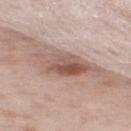Clinical impression: The lesion was photographed on a routine skin check and not biopsied; there is no pathology result.